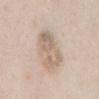- follow-up: imaged on a skin check; not biopsied
- subject: female, in their 40s
- illumination: white-light illumination
- site: the mid back
- imaging modality: ~15 mm crop, total-body skin-cancer survey